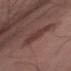Q: Was a biopsy performed?
A: total-body-photography surveillance lesion; no biopsy
Q: What is the anatomic site?
A: the right upper arm
Q: Illumination type?
A: white-light illumination
Q: Who is the patient?
A: male, aged 48 to 52
Q: What did automated image analysis measure?
A: a border-irregularity index near 3.5/10 and radial color variation of about 0.5; a classifier nevus-likeness of about 50/100 and a lesion-detection confidence of about 100/100
Q: What kind of image is this?
A: total-body-photography crop, ~15 mm field of view